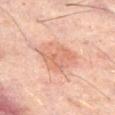Notes:
• workup: no biopsy performed (imaged during a skin exam)
• patient: male, aged 58–62
• automated metrics: a lesion color around L≈62 a*≈23 b*≈30 in CIELAB, a lesion–skin lightness drop of about 8, and a normalized lesion–skin contrast near 5.5; lesion-presence confidence of about 100/100
• body site: the front of the torso
• lesion diameter: ~5 mm (longest diameter)
• image: ~15 mm crop, total-body skin-cancer survey
• illumination: cross-polarized illumination